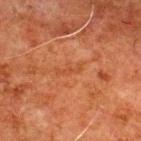body site: the upper back | patient: male, aged 78 to 82 | acquisition: ~15 mm tile from a whole-body skin photo | automated metrics: an eccentricity of roughly 0.95 and a shape-asymmetry score of about 0.5 (0 = symmetric); a lesion color around L≈39 a*≈24 b*≈34 in CIELAB and roughly 5 lightness units darker than nearby skin; a detector confidence of about 95 out of 100 that the crop contains a lesion.